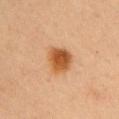The lesion was photographed on a routine skin check and not biopsied; there is no pathology result. A female patient, aged 63–67. Imaged with cross-polarized lighting. An algorithmic analysis of the crop reported an average lesion color of about L≈47 a*≈22 b*≈36 (CIELAB) and a normalized border contrast of about 10. And it measured a border-irregularity index near 2/10, a within-lesion color-variation index near 4.5/10, and a peripheral color-asymmetry measure near 1.5. Longest diameter approximately 3.5 mm. The lesion is located on the arm. Cropped from a total-body skin-imaging series; the visible field is about 15 mm.